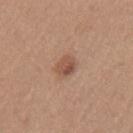The lesion was tiled from a total-body skin photograph and was not biopsied. The tile uses white-light illumination. From the mid back. This image is a 15 mm lesion crop taken from a total-body photograph. The lesion's longest dimension is about 2.5 mm. The patient is a female aged around 35. The lesion-visualizer software estimated a nevus-likeness score of about 75/100 and lesion-presence confidence of about 100/100.